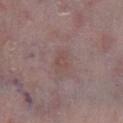Notes:
– biopsy status · total-body-photography surveillance lesion; no biopsy
– patient · male, about 65 years old
– lighting · white-light illumination
– location · the right lower leg
– imaging modality · total-body-photography crop, ~15 mm field of view